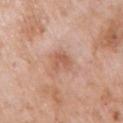Notes:
• workup · no biopsy performed (imaged during a skin exam)
• lesion size · ≈3 mm
• tile lighting · white-light
• automated metrics · an area of roughly 5 mm² and two-axis asymmetry of about 0.3
• imaging modality · ~15 mm tile from a whole-body skin photo
• patient · female, roughly 70 years of age
• site · the right upper arm